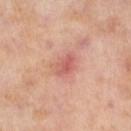Impression: Part of a total-body skin-imaging series; this lesion was reviewed on a skin check and was not flagged for biopsy. Clinical summary: Imaged with cross-polarized lighting. On the left lower leg. A female patient, aged 53 to 57. The recorded lesion diameter is about 3 mm. The lesion-visualizer software estimated a lesion color around L≈60 a*≈29 b*≈27 in CIELAB, roughly 9 lightness units darker than nearby skin, and a normalized border contrast of about 6. A 15 mm crop from a total-body photograph taken for skin-cancer surveillance.